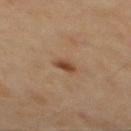* site · the mid back
* tile lighting · cross-polarized
* diameter · about 2 mm
* image · 15 mm crop, total-body photography
* automated lesion analysis · a border-irregularity rating of about 2/10; a nevus-likeness score of about 95/100
* subject · male, approximately 70 years of age